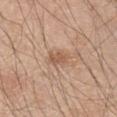Impression:
This lesion was catalogued during total-body skin photography and was not selected for biopsy.
Context:
The subject is a male aged around 45. The tile uses white-light illumination. The total-body-photography lesion software estimated a lesion–skin lightness drop of about 9 and a normalized border contrast of about 6.5. The analysis additionally found a border-irregularity rating of about 3/10, a color-variation rating of about 1/10, and radial color variation of about 0.5. Measured at roughly 3 mm in maximum diameter. A lesion tile, about 15 mm wide, cut from a 3D total-body photograph. On the right upper arm.